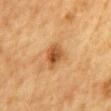Impression:
Imaged during a routine full-body skin examination; the lesion was not biopsied and no histopathology is available.
Clinical summary:
A roughly 15 mm field-of-view crop from a total-body skin photograph. Automated tile analysis of the lesion measured a lesion area of about 7 mm² and an outline eccentricity of about 0.65 (0 = round, 1 = elongated). And it measured a border-irregularity index near 2/10 and a peripheral color-asymmetry measure near 2. A male patient in their mid-80s or older. This is a cross-polarized tile. Longest diameter approximately 3.5 mm. On the chest.